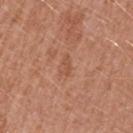Q: Is there a histopathology result?
A: imaged on a skin check; not biopsied
Q: How was the tile lit?
A: white-light illumination
Q: Where on the body is the lesion?
A: the left upper arm
Q: Lesion size?
A: ≈2.5 mm
Q: What kind of image is this?
A: ~15 mm tile from a whole-body skin photo
Q: What did automated image analysis measure?
A: a border-irregularity index near 4.5/10, internal color variation of about 0 on a 0–10 scale, and radial color variation of about 0; a nevus-likeness score of about 0/100
Q: Patient demographics?
A: female, aged around 40